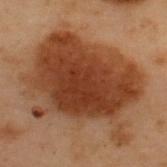Impression:
The lesion was tiled from a total-body skin photograph and was not biopsied.
Context:
A 15 mm close-up tile from a total-body photography series done for melanoma screening. The lesion's longest dimension is about 11.5 mm. A male subject, about 55 years old. On the upper back. This is a cross-polarized tile.